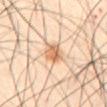<record>
  <biopsy_status>not biopsied; imaged during a skin examination</biopsy_status>
  <site>front of the torso</site>
  <image>
    <source>total-body photography crop</source>
    <field_of_view_mm>15</field_of_view_mm>
  </image>
  <lighting>cross-polarized</lighting>
  <patient>
    <sex>male</sex>
    <age_approx>50</age_approx>
  </patient>
</record>